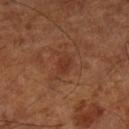Findings:
- image-analysis metrics: an area of roughly 3.5 mm², a shape eccentricity near 0.8, and a symmetry-axis asymmetry near 0.3; a classifier nevus-likeness of about 0/100 and lesion-presence confidence of about 100/100
- location: the left lower leg
- patient: aged around 65
- image source: 15 mm crop, total-body photography
- lesion diameter: about 2.5 mm